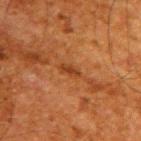* follow-up: imaged on a skin check; not biopsied
* diameter: ≈2.5 mm
* illumination: cross-polarized illumination
* subject: male, in their 60s
* image: ~15 mm crop, total-body skin-cancer survey
* anatomic site: the upper back